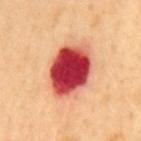Image and clinical context: A male patient aged 48 to 52. On the mid back. Measured at roughly 7 mm in maximum diameter. A 15 mm close-up extracted from a 3D total-body photography capture. The lesion-visualizer software estimated a mean CIELAB color near L≈50 a*≈41 b*≈32, roughly 25 lightness units darker than nearby skin, and a normalized lesion–skin contrast near 16. And it measured a border-irregularity index near 1.5/10 and a peripheral color-asymmetry measure near 3. The software also gave a nevus-likeness score of about 0/100. This is a cross-polarized tile.